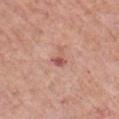Captured during whole-body skin photography for melanoma surveillance; the lesion was not biopsied. This image is a 15 mm lesion crop taken from a total-body photograph. Located on the chest. The subject is a male aged approximately 55. The tile uses white-light illumination. Measured at roughly 2.5 mm in maximum diameter. Automated tile analysis of the lesion measured an area of roughly 3 mm², an eccentricity of roughly 0.75, and a shape-asymmetry score of about 0.55 (0 = symmetric).